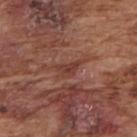{"biopsy_status": "not biopsied; imaged during a skin examination", "patient": {"sex": "male", "age_approx": 75}, "image": {"source": "total-body photography crop", "field_of_view_mm": 15}, "automated_metrics": {"area_mm2_approx": 3.5, "shape_asymmetry": 0.45, "cielab_L": 39, "cielab_a": 25, "cielab_b": 25, "vs_skin_darker_L": 8.0, "vs_skin_contrast_norm": 6.5, "border_irregularity_0_10": 4.5, "color_variation_0_10": 2.5, "nevus_likeness_0_100": 0, "lesion_detection_confidence_0_100": 80}, "site": "right upper arm", "lesion_size": {"long_diameter_mm_approx": 3.0}}